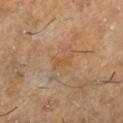{"patient": {"sex": "male", "age_approx": 60}, "site": "left lower leg", "automated_metrics": {"area_mm2_approx": 4.0, "eccentricity": 0.8, "shape_asymmetry": 0.4, "cielab_L": 49, "cielab_a": 18, "cielab_b": 35, "vs_skin_darker_L": 5.0}, "lighting": "cross-polarized", "image": {"source": "total-body photography crop", "field_of_view_mm": 15}, "lesion_size": {"long_diameter_mm_approx": 3.0}}